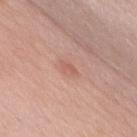Q: Is there a histopathology result?
A: no biopsy performed (imaged during a skin exam)
Q: What lighting was used for the tile?
A: white-light
Q: What kind of image is this?
A: ~15 mm crop, total-body skin-cancer survey
Q: Lesion location?
A: the chest
Q: What are the patient's age and sex?
A: male, in their 60s
Q: What did automated image analysis measure?
A: an eccentricity of roughly 0.95 and two-axis asymmetry of about 0.5; a border-irregularity index near 5.5/10 and internal color variation of about 0 on a 0–10 scale